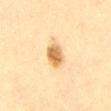acquisition: 15 mm crop, total-body photography
patient: male, aged 33–37
tile lighting: cross-polarized
anatomic site: the abdomen